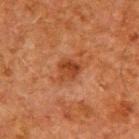Q: Is there a histopathology result?
A: imaged on a skin check; not biopsied